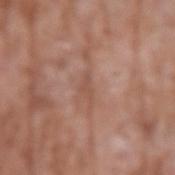Impression:
Captured during whole-body skin photography for melanoma surveillance; the lesion was not biopsied.
Background:
This is a white-light tile. A 15 mm close-up extracted from a 3D total-body photography capture. A male subject aged 58 to 62. The lesion is on the arm. The total-body-photography lesion software estimated a footprint of about 2.5 mm². And it measured a lesion color around L≈51 a*≈21 b*≈28 in CIELAB and a normalized lesion–skin contrast near 5.